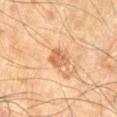Impression:
The lesion was photographed on a routine skin check and not biopsied; there is no pathology result.
Context:
This is a cross-polarized tile. A male patient aged 68 to 72. This image is a 15 mm lesion crop taken from a total-body photograph. Measured at roughly 2.5 mm in maximum diameter. On the left thigh.